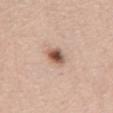Clinical impression: No biopsy was performed on this lesion — it was imaged during a full skin examination and was not determined to be concerning. Background: On the back. The tile uses white-light illumination. The subject is a male about 60 years old. The recorded lesion diameter is about 3 mm. A 15 mm crop from a total-body photograph taken for skin-cancer surveillance.